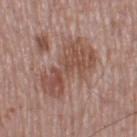{"biopsy_status": "not biopsied; imaged during a skin examination", "automated_metrics": {"cielab_L": 50, "cielab_a": 20, "cielab_b": 26, "vs_skin_darker_L": 9.0, "lesion_detection_confidence_0_100": 100}, "lesion_size": {"long_diameter_mm_approx": 7.5}, "site": "left thigh", "lighting": "white-light", "image": {"source": "total-body photography crop", "field_of_view_mm": 15}, "patient": {"sex": "male", "age_approx": 55}}